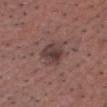biopsy status = total-body-photography surveillance lesion; no biopsy | patient = male, aged around 55 | image source = ~15 mm tile from a whole-body skin photo | anatomic site = the head or neck | tile lighting = white-light | automated lesion analysis = a normalized lesion–skin contrast near 7.5; a border-irregularity rating of about 2/10, a color-variation rating of about 5/10, and peripheral color asymmetry of about 1.5; an automated nevus-likeness rating near 75 out of 100.